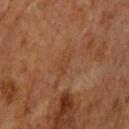biopsy_status: not biopsied; imaged during a skin examination
lighting: cross-polarized
patient:
  sex: male
  age_approx: 65
site: left upper arm
image:
  source: total-body photography crop
  field_of_view_mm: 15
lesion_size:
  long_diameter_mm_approx: 3.0